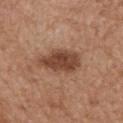follow-up: catalogued during a skin exam; not biopsied
acquisition: total-body-photography crop, ~15 mm field of view
diameter: ~5.5 mm (longest diameter)
tile lighting: white-light illumination
patient: female, aged 78–82
location: the mid back